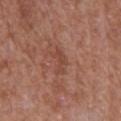Q: Was a biopsy performed?
A: imaged on a skin check; not biopsied
Q: How was this image acquired?
A: 15 mm crop, total-body photography
Q: Who is the patient?
A: male, aged around 65
Q: Where on the body is the lesion?
A: the front of the torso
Q: How was the tile lit?
A: white-light illumination